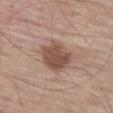Clinical impression: Imaged during a routine full-body skin examination; the lesion was not biopsied and no histopathology is available. Context: A 15 mm close-up extracted from a 3D total-body photography capture. A male patient aged 63–67. Approximately 4 mm at its widest. An algorithmic analysis of the crop reported an automated nevus-likeness rating near 15 out of 100 and lesion-presence confidence of about 100/100. On the left thigh.